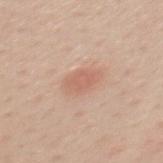Image and clinical context: The lesion's longest dimension is about 4 mm. A roughly 15 mm field-of-view crop from a total-body skin photograph. The total-body-photography lesion software estimated a lesion–skin lightness drop of about 8 and a normalized lesion–skin contrast near 5.5. The analysis additionally found a border-irregularity rating of about 1.5/10, a color-variation rating of about 2/10, and peripheral color asymmetry of about 0.5. The software also gave an automated nevus-likeness rating near 55 out of 100 and a lesion-detection confidence of about 100/100. Located on the mid back. A female subject, in their 40s. Imaged with white-light lighting.